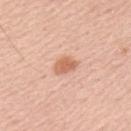  biopsy_status: not biopsied; imaged during a skin examination
  patient:
    sex: male
    age_approx: 65
  site: left upper arm
  image:
    source: total-body photography crop
    field_of_view_mm: 15